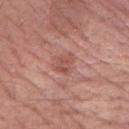<record>
<biopsy_status>not biopsied; imaged during a skin examination</biopsy_status>
<site>right forearm</site>
<automated_metrics>
  <border_irregularity_0_10>3.5</border_irregularity_0_10>
  <color_variation_0_10>3.0</color_variation_0_10>
</automated_metrics>
<patient>
  <sex>male</sex>
  <age_approx>50</age_approx>
</patient>
<image>
  <source>total-body photography crop</source>
  <field_of_view_mm>15</field_of_view_mm>
</image>
</record>